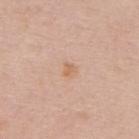body site: the back
lesion size: ≈1.5 mm
subject: female, roughly 50 years of age
illumination: white-light illumination
TBP lesion metrics: an area of roughly 1.5 mm², an outline eccentricity of about 0.55 (0 = round, 1 = elongated), and a symmetry-axis asymmetry near 0.25; a lesion color around L≈63 a*≈20 b*≈33 in CIELAB, roughly 7 lightness units darker than nearby skin, and a lesion-to-skin contrast of about 6 (normalized; higher = more distinct); a within-lesion color-variation index near 0/10 and peripheral color asymmetry of about 0; lesion-presence confidence of about 100/100
image source: ~15 mm crop, total-body skin-cancer survey